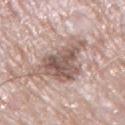Clinical impression: No biopsy was performed on this lesion — it was imaged during a full skin examination and was not determined to be concerning. Background: Cropped from a whole-body photographic skin survey; the tile spans about 15 mm. The lesion is located on the right upper arm. A male subject approximately 65 years of age.